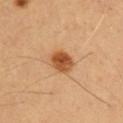Imaged during a routine full-body skin examination; the lesion was not biopsied and no histopathology is available. The total-body-photography lesion software estimated a lesion color around L≈50 a*≈24 b*≈39 in CIELAB and a normalized lesion–skin contrast near 10. The software also gave a nevus-likeness score of about 100/100 and lesion-presence confidence of about 100/100. On the chest. A male patient aged approximately 55. A lesion tile, about 15 mm wide, cut from a 3D total-body photograph. Measured at roughly 3.5 mm in maximum diameter. Imaged with cross-polarized lighting.